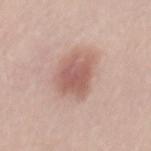{
  "patient": {
    "sex": "male",
    "age_approx": 30
  },
  "image": {
    "source": "total-body photography crop",
    "field_of_view_mm": 15
  }
}